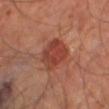Assessment: This lesion was catalogued during total-body skin photography and was not selected for biopsy. Clinical summary: A male subject, aged around 65. A 15 mm close-up extracted from a 3D total-body photography capture. Captured under cross-polarized illumination. The lesion is on the right thigh. Measured at roughly 4.5 mm in maximum diameter.